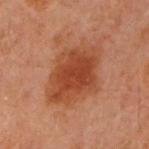This lesion was catalogued during total-body skin photography and was not selected for biopsy.
The lesion is located on the left arm.
Measured at roughly 7.5 mm in maximum diameter.
A female patient aged 58–62.
Cropped from a total-body skin-imaging series; the visible field is about 15 mm.
Captured under cross-polarized illumination.
Automated image analysis of the tile measured an area of roughly 27 mm², an outline eccentricity of about 0.75 (0 = round, 1 = elongated), and a shape-asymmetry score of about 0.2 (0 = symmetric). It also reported a border-irregularity rating of about 2.5/10.